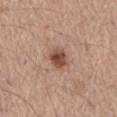No biopsy was performed on this lesion — it was imaged during a full skin examination and was not determined to be concerning. The tile uses white-light illumination. The lesion is located on the abdomen. A 15 mm crop from a total-body photograph taken for skin-cancer surveillance. A male subject, aged approximately 65. The total-body-photography lesion software estimated a footprint of about 5.5 mm² and an outline eccentricity of about 0.55 (0 = round, 1 = elongated). It also reported a mean CIELAB color near L≈48 a*≈21 b*≈27, a lesion–skin lightness drop of about 14, and a normalized lesion–skin contrast near 10. It also reported a lesion-detection confidence of about 100/100. The recorded lesion diameter is about 3 mm.